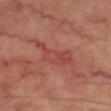notes — imaged on a skin check; not biopsied | image source — 15 mm crop, total-body photography | subject — male, roughly 70 years of age | image-analysis metrics — a footprint of about 9.5 mm², an outline eccentricity of about 0.9 (0 = round, 1 = elongated), and a shape-asymmetry score of about 0.5 (0 = symmetric); a mean CIELAB color near L≈44 a*≈29 b*≈25, about 6 CIELAB-L* units darker than the surrounding skin, and a normalized lesion–skin contrast near 5; border irregularity of about 6 on a 0–10 scale and a within-lesion color-variation index near 3.5/10; a classifier nevus-likeness of about 0/100 | anatomic site — the left thigh.